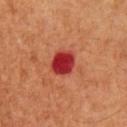Imaged during a routine full-body skin examination; the lesion was not biopsied and no histopathology is available. The lesion is located on the upper back. Longest diameter approximately 3 mm. The lesion-visualizer software estimated an average lesion color of about L≈34 a*≈41 b*≈29 (CIELAB), a lesion–skin lightness drop of about 16, and a normalized border contrast of about 13. And it measured a border-irregularity rating of about 1.5/10 and radial color variation of about 1. A lesion tile, about 15 mm wide, cut from a 3D total-body photograph. A male patient about 60 years old. Captured under cross-polarized illumination.